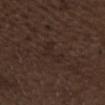workup: catalogued during a skin exam; not biopsied
imaging modality: 15 mm crop, total-body photography
diameter: ≈3 mm
subject: male, aged approximately 70
location: the head or neck
tile lighting: white-light